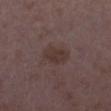– follow-up · total-body-photography surveillance lesion; no biopsy
– location · the leg
– image source · ~15 mm crop, total-body skin-cancer survey
– subject · female, aged around 35
– lesion diameter · ≈3.5 mm
– TBP lesion metrics · border irregularity of about 2 on a 0–10 scale, internal color variation of about 2 on a 0–10 scale, and a peripheral color-asymmetry measure near 0.5; a nevus-likeness score of about 5/100